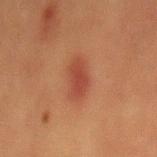Assessment:
No biopsy was performed on this lesion — it was imaged during a full skin examination and was not determined to be concerning.
Background:
Measured at roughly 4 mm in maximum diameter. Cropped from a total-body skin-imaging series; the visible field is about 15 mm. Captured under cross-polarized illumination. From the mid back. A male patient, aged around 40.